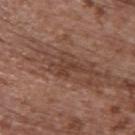Captured during whole-body skin photography for melanoma surveillance; the lesion was not biopsied. The lesion is on the upper back. An algorithmic analysis of the crop reported an area of roughly 5 mm² and an eccentricity of roughly 0.85. It also reported a mean CIELAB color near L≈40 a*≈19 b*≈26, a lesion–skin lightness drop of about 8, and a normalized border contrast of about 7. It also reported a border-irregularity rating of about 7/10, a color-variation rating of about 2/10, and radial color variation of about 0.5. Longest diameter approximately 4 mm. The subject is a female in their mid- to late 60s. A 15 mm crop from a total-body photograph taken for skin-cancer surveillance.